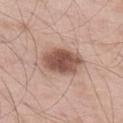This lesion was catalogued during total-body skin photography and was not selected for biopsy. A close-up tile cropped from a whole-body skin photograph, about 15 mm across. The patient is a male aged approximately 55. Imaged with white-light lighting. Approximately 5 mm at its widest. Automated image analysis of the tile measured a lesion area of about 14 mm² and an eccentricity of roughly 0.75. And it measured a lesion color around L≈53 a*≈21 b*≈26 in CIELAB, a lesion–skin lightness drop of about 15, and a normalized lesion–skin contrast near 10. The analysis additionally found a border-irregularity index near 1.5/10, a color-variation rating of about 4/10, and a peripheral color-asymmetry measure near 1. The analysis additionally found a classifier nevus-likeness of about 90/100 and a detector confidence of about 100 out of 100 that the crop contains a lesion. Located on the abdomen.